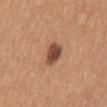Recorded during total-body skin imaging; not selected for excision or biopsy. Located on the back. The subject is a female approximately 40 years of age. A roughly 15 mm field-of-view crop from a total-body skin photograph.